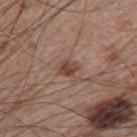<case>
<biopsy_status>not biopsied; imaged during a skin examination</biopsy_status>
<patient>
  <sex>male</sex>
  <age_approx>65</age_approx>
</patient>
<image>
  <source>total-body photography crop</source>
  <field_of_view_mm>15</field_of_view_mm>
</image>
<site>left upper arm</site>
</case>